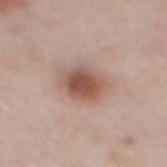Clinical impression:
Captured during whole-body skin photography for melanoma surveillance; the lesion was not biopsied.
Acquisition and patient details:
The patient is a female aged 28 to 32. An algorithmic analysis of the crop reported a mean CIELAB color near L≈53 a*≈21 b*≈26 and a normalized lesion–skin contrast near 9. The analysis additionally found a border-irregularity rating of about 2/10, a color-variation rating of about 4/10, and radial color variation of about 1. A roughly 15 mm field-of-view crop from a total-body skin photograph. The lesion's longest dimension is about 4.5 mm. On the mid back. This is a white-light tile.